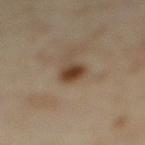Clinical summary:
A female patient, aged approximately 35. From the right lower leg. A 15 mm close-up extracted from a 3D total-body photography capture. Imaged with cross-polarized lighting.